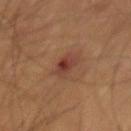Captured during whole-body skin photography for melanoma surveillance; the lesion was not biopsied. A male subject, in their mid- to late 60s. Measured at roughly 2.5 mm in maximum diameter. A close-up tile cropped from a whole-body skin photograph, about 15 mm across. This is a cross-polarized tile. Automated image analysis of the tile measured a footprint of about 5 mm², an outline eccentricity of about 0.5 (0 = round, 1 = elongated), and a shape-asymmetry score of about 0.2 (0 = symmetric). The software also gave a border-irregularity rating of about 2/10. The lesion is on the mid back.